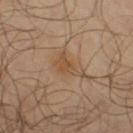Impression: The lesion was tiled from a total-body skin photograph and was not biopsied. Acquisition and patient details: A male patient in their mid- to late 60s. The lesion is on the leg. This image is a 15 mm lesion crop taken from a total-body photograph.